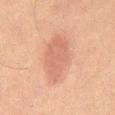Recorded during total-body skin imaging; not selected for excision or biopsy.
This is a cross-polarized tile.
A 15 mm close-up tile from a total-body photography series done for melanoma screening.
The recorded lesion diameter is about 6 mm.
On the mid back.
A male patient, in their mid- to late 60s.